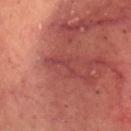notes — catalogued during a skin exam; not biopsied
TBP lesion metrics — a lesion area of about 3 mm² and a shape eccentricity near 0.9; about 6 CIELAB-L* units darker than the surrounding skin and a normalized border contrast of about 5; a border-irregularity index near 8.5/10 and internal color variation of about 0 on a 0–10 scale; a classifier nevus-likeness of about 0/100 and lesion-presence confidence of about 50/100
location — the head or neck
imaging modality — ~15 mm tile from a whole-body skin photo
lesion diameter — ~3 mm (longest diameter)
patient — male, aged 63 to 67
illumination — cross-polarized illumination